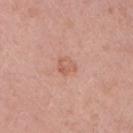Q: Was this lesion biopsied?
A: total-body-photography surveillance lesion; no biopsy
Q: How was this image acquired?
A: 15 mm crop, total-body photography
Q: What is the anatomic site?
A: the right upper arm
Q: How large is the lesion?
A: ~2.5 mm (longest diameter)
Q: What are the patient's age and sex?
A: female, in their 40s
Q: How was the tile lit?
A: white-light illumination